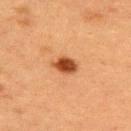{"biopsy_status": "not biopsied; imaged during a skin examination", "site": "upper back", "lighting": "cross-polarized", "image": {"source": "total-body photography crop", "field_of_view_mm": 15}, "lesion_size": {"long_diameter_mm_approx": 3.0}, "patient": {"sex": "female", "age_approx": 55}}